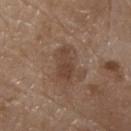biopsy_status: not biopsied; imaged during a skin examination
lighting: white-light
site: front of the torso
patient:
  sex: male
  age_approx: 80
image:
  source: total-body photography crop
  field_of_view_mm: 15
automated_metrics:
  eccentricity: 0.9